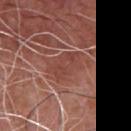{
  "biopsy_status": "not biopsied; imaged during a skin examination",
  "lighting": "white-light",
  "automated_metrics": {
    "area_mm2_approx": 9.0,
    "eccentricity": 0.8,
    "shape_asymmetry": 0.25,
    "border_irregularity_0_10": 3.0,
    "color_variation_0_10": 2.5,
    "peripheral_color_asymmetry": 1.0,
    "nevus_likeness_0_100": 0,
    "lesion_detection_confidence_0_100": 60
  },
  "patient": {
    "sex": "male",
    "age_approx": 75
  },
  "image": {
    "source": "total-body photography crop",
    "field_of_view_mm": 15
  },
  "site": "chest",
  "lesion_size": {
    "long_diameter_mm_approx": 4.0
  }
}